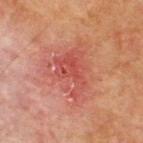Q: Is there a histopathology result?
A: imaged on a skin check; not biopsied
Q: Automated lesion metrics?
A: a footprint of about 20 mm², a shape eccentricity near 0.65, and two-axis asymmetry of about 0.5; an average lesion color of about L≈43 a*≈27 b*≈25 (CIELAB) and a normalized lesion–skin contrast near 5.5; a border-irregularity rating of about 6.5/10, internal color variation of about 5 on a 0–10 scale, and peripheral color asymmetry of about 1.5
Q: Where on the body is the lesion?
A: the upper back
Q: What kind of image is this?
A: ~15 mm crop, total-body skin-cancer survey
Q: How was the tile lit?
A: cross-polarized illumination
Q: Patient demographics?
A: male, aged approximately 70
Q: How large is the lesion?
A: ≈7 mm